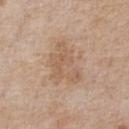{
  "biopsy_status": "not biopsied; imaged during a skin examination",
  "patient": {
    "sex": "male",
    "age_approx": 65
  },
  "automated_metrics": {
    "area_mm2_approx": 16.0,
    "eccentricity": 0.7,
    "shape_asymmetry": 0.35,
    "color_variation_0_10": 3.0,
    "peripheral_color_asymmetry": 1.0,
    "lesion_detection_confidence_0_100": 100
  },
  "site": "chest",
  "image": {
    "source": "total-body photography crop",
    "field_of_view_mm": 15
  },
  "lighting": "white-light"
}